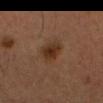Clinical impression: No biopsy was performed on this lesion — it was imaged during a full skin examination and was not determined to be concerning. Acquisition and patient details: A 15 mm close-up tile from a total-body photography series done for melanoma screening. Located on the head or neck. A male patient, approximately 35 years of age. The recorded lesion diameter is about 3 mm. Automated tile analysis of the lesion measured an average lesion color of about L≈27 a*≈17 b*≈26 (CIELAB) and a normalized border contrast of about 9. The analysis additionally found a color-variation rating of about 3/10 and radial color variation of about 1.